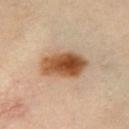Findings:
* notes — total-body-photography surveillance lesion; no biopsy
* size — ≈5.5 mm
* TBP lesion metrics — an average lesion color of about L≈51 a*≈20 b*≈35 (CIELAB), about 16 CIELAB-L* units darker than the surrounding skin, and a normalized lesion–skin contrast near 12; a nevus-likeness score of about 100/100 and lesion-presence confidence of about 100/100
* tile lighting — cross-polarized
* patient — female, aged 33 to 37
* imaging modality — ~15 mm crop, total-body skin-cancer survey
* anatomic site — the chest